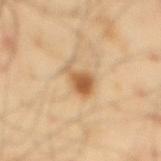Clinical impression:
The lesion was tiled from a total-body skin photograph and was not biopsied.
Context:
Located on the mid back. This image is a 15 mm lesion crop taken from a total-body photograph. The recorded lesion diameter is about 4 mm. The patient is a male about 40 years old.